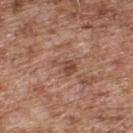workup: no biopsy performed (imaged during a skin exam) | size: ≈3 mm | location: the back | subject: male, aged approximately 55 | illumination: white-light illumination | imaging modality: total-body-photography crop, ~15 mm field of view.